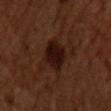The lesion was photographed on a routine skin check and not biopsied; there is no pathology result.